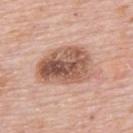Clinical impression:
Imaged during a routine full-body skin examination; the lesion was not biopsied and no histopathology is available.